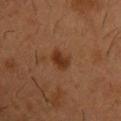Clinical impression: The lesion was photographed on a routine skin check and not biopsied; there is no pathology result. Background: The patient is a male approximately 55 years of age. The lesion is located on the chest. The lesion's longest dimension is about 2.5 mm. Imaged with cross-polarized lighting. This image is a 15 mm lesion crop taken from a total-body photograph.